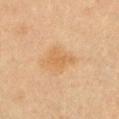Imaged during a routine full-body skin examination; the lesion was not biopsied and no histopathology is available.
On the upper back.
A male subject aged 63 to 67.
The lesion's longest dimension is about 3.5 mm.
Captured under cross-polarized illumination.
A 15 mm crop from a total-body photograph taken for skin-cancer surveillance.